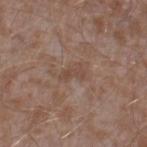site: leg
lesion_size:
  long_diameter_mm_approx: 3.0
lighting: white-light
image:
  source: total-body photography crop
  field_of_view_mm: 15
patient:
  sex: male
  age_approx: 45